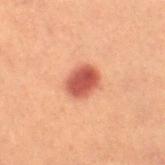This lesion was catalogued during total-body skin photography and was not selected for biopsy.